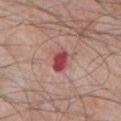Captured during whole-body skin photography for melanoma surveillance; the lesion was not biopsied.
The lesion is located on the chest.
Automated image analysis of the tile measured a lesion color around L≈47 a*≈33 b*≈22 in CIELAB.
The lesion's longest dimension is about 3 mm.
A roughly 15 mm field-of-view crop from a total-body skin photograph.
The subject is a male aged 78–82.